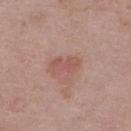Clinical impression:
Recorded during total-body skin imaging; not selected for excision or biopsy.
Background:
The lesion is located on the right lower leg. Approximately 3.5 mm at its widest. An algorithmic analysis of the crop reported a footprint of about 6 mm², an outline eccentricity of about 0.85 (0 = round, 1 = elongated), and a shape-asymmetry score of about 0.3 (0 = symmetric). The software also gave an automated nevus-likeness rating near 30 out of 100 and a detector confidence of about 100 out of 100 that the crop contains a lesion. A female patient, in their mid- to late 60s. Captured under white-light illumination. Cropped from a total-body skin-imaging series; the visible field is about 15 mm.